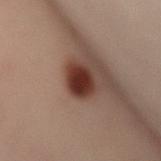This image is a 15 mm lesion crop taken from a total-body photograph.
Imaged with cross-polarized lighting.
An algorithmic analysis of the crop reported a footprint of about 7 mm². It also reported a lesion color around L≈32 a*≈22 b*≈24 in CIELAB and a normalized border contrast of about 14.5. The software also gave a border-irregularity rating of about 2/10, a color-variation rating of about 3/10, and a peripheral color-asymmetry measure near 1.
Longest diameter approximately 4 mm.
From the mid back.
A female subject, aged 38–42.